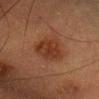workup = imaged on a skin check; not biopsied | lighting = cross-polarized illumination | patient = female, in their mid- to late 50s | acquisition = 15 mm crop, total-body photography | site = the head or neck | diameter = ~5 mm (longest diameter) | image-analysis metrics = an average lesion color of about L≈29 a*≈20 b*≈26 (CIELAB), about 7 CIELAB-L* units darker than the surrounding skin, and a normalized lesion–skin contrast near 7.5; border irregularity of about 2.5 on a 0–10 scale and a within-lesion color-variation index near 2.5/10.